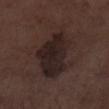Recorded during total-body skin imaging; not selected for excision or biopsy. Cropped from a total-body skin-imaging series; the visible field is about 15 mm. This is a white-light tile. Located on the left lower leg. Approximately 7.5 mm at its widest. A male subject aged approximately 70.